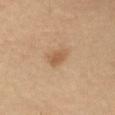{"lesion_size": {"long_diameter_mm_approx": 2.5}, "image": {"source": "total-body photography crop", "field_of_view_mm": 15}, "site": "right upper arm", "patient": {"sex": "male", "age_approx": 60}}